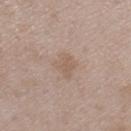| key | value |
|---|---|
| biopsy status | no biopsy performed (imaged during a skin exam) |
| image | 15 mm crop, total-body photography |
| illumination | white-light illumination |
| automated metrics | internal color variation of about 1.5 on a 0–10 scale; a detector confidence of about 100 out of 100 that the crop contains a lesion |
| site | the left thigh |
| lesion diameter | about 2.5 mm |
| patient | male, aged approximately 50 |